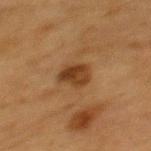notes = no biopsy performed (imaged during a skin exam); illumination = cross-polarized; image source = total-body-photography crop, ~15 mm field of view; size = ~3.5 mm (longest diameter); body site = the upper back; subject = female, in their mid- to late 50s.